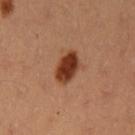Q: Was a biopsy performed?
A: total-body-photography surveillance lesion; no biopsy
Q: Who is the patient?
A: female, aged around 35
Q: What kind of image is this?
A: ~15 mm crop, total-body skin-cancer survey
Q: Illumination type?
A: cross-polarized illumination
Q: Lesion location?
A: the left upper arm
Q: Automated lesion metrics?
A: a mean CIELAB color near L≈38 a*≈26 b*≈33 and roughly 16 lightness units darker than nearby skin; border irregularity of about 1.5 on a 0–10 scale, a color-variation rating of about 3.5/10, and a peripheral color-asymmetry measure near 1; a classifier nevus-likeness of about 100/100 and lesion-presence confidence of about 100/100
Q: How large is the lesion?
A: ~3.5 mm (longest diameter)